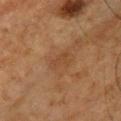| feature | finding |
|---|---|
| notes | total-body-photography surveillance lesion; no biopsy |
| illumination | cross-polarized |
| diameter | ≈3 mm |
| subject | male, aged 58 to 62 |
| image-analysis metrics | an area of roughly 4 mm², an outline eccentricity of about 0.85 (0 = round, 1 = elongated), and a shape-asymmetry score of about 0.35 (0 = symmetric) |
| location | the chest |
| imaging modality | 15 mm crop, total-body photography |